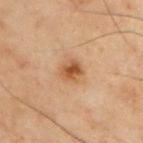Q: Was a biopsy performed?
A: catalogued during a skin exam; not biopsied
Q: Illumination type?
A: cross-polarized illumination
Q: How was this image acquired?
A: ~15 mm crop, total-body skin-cancer survey
Q: Lesion location?
A: the chest
Q: Automated lesion metrics?
A: a lesion area of about 5.5 mm², an eccentricity of roughly 0.25, and a shape-asymmetry score of about 0.2 (0 = symmetric); about 10 CIELAB-L* units darker than the surrounding skin and a lesion-to-skin contrast of about 8.5 (normalized; higher = more distinct); border irregularity of about 1.5 on a 0–10 scale, a color-variation rating of about 4.5/10, and radial color variation of about 1.5
Q: How large is the lesion?
A: about 2.5 mm
Q: What are the patient's age and sex?
A: male, aged 53 to 57